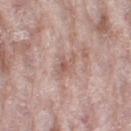biopsy_status: not biopsied; imaged during a skin examination
image:
  source: total-body photography crop
  field_of_view_mm: 15
site: leg
patient:
  sex: female
  age_approx: 70
lesion_size:
  long_diameter_mm_approx: 3.5